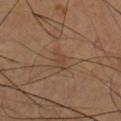The lesion was photographed on a routine skin check and not biopsied; there is no pathology result.
About 2.5 mm across.
A lesion tile, about 15 mm wide, cut from a 3D total-body photograph.
From the chest.
Captured under cross-polarized illumination.
A male patient, aged around 40.